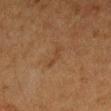biopsy_status: not biopsied; imaged during a skin examination
patient:
  sex: female
  age_approx: 55
site: left forearm
automated_metrics:
  cielab_L: 34
  cielab_a: 17
  cielab_b: 29
  vs_skin_darker_L: 4.0
  vs_skin_contrast_norm: 4.5
lesion_size:
  long_diameter_mm_approx: 3.0
lighting: cross-polarized
image:
  source: total-body photography crop
  field_of_view_mm: 15